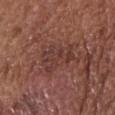The tile uses white-light illumination.
From the chest.
A male subject, aged approximately 75.
Longest diameter approximately 5 mm.
Cropped from a whole-body photographic skin survey; the tile spans about 15 mm.